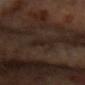biopsy status: total-body-photography surveillance lesion; no biopsy | anatomic site: the right forearm | TBP lesion metrics: a border-irregularity rating of about 4/10 and a peripheral color-asymmetry measure near 0.5; a classifier nevus-likeness of about 0/100 and a detector confidence of about 65 out of 100 that the crop contains a lesion | lighting: cross-polarized illumination | image: ~15 mm crop, total-body skin-cancer survey | patient: female, aged approximately 60 | diameter: ≈3 mm.